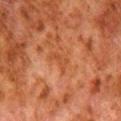A lesion tile, about 15 mm wide, cut from a 3D total-body photograph. A male subject about 80 years old. Captured under cross-polarized illumination. From the leg.